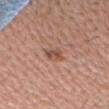Findings:
* biopsy status · total-body-photography surveillance lesion; no biopsy
* patient · male, approximately 50 years of age
* location · the head or neck
* diameter · about 2.5 mm
* TBP lesion metrics · a normalized border contrast of about 7; internal color variation of about 2.5 on a 0–10 scale and radial color variation of about 1
* image · total-body-photography crop, ~15 mm field of view
* lighting · white-light illumination